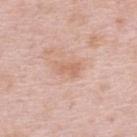Q: Is there a histopathology result?
A: catalogued during a skin exam; not biopsied
Q: What are the patient's age and sex?
A: female, roughly 65 years of age
Q: How was this image acquired?
A: ~15 mm crop, total-body skin-cancer survey
Q: What did automated image analysis measure?
A: roughly 7 lightness units darker than nearby skin and a lesion-to-skin contrast of about 5.5 (normalized; higher = more distinct); a border-irregularity index near 3/10 and a peripheral color-asymmetry measure near 0.5; an automated nevus-likeness rating near 0 out of 100
Q: Lesion location?
A: the upper back
Q: Lesion size?
A: ≈3 mm